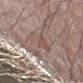notes = catalogued during a skin exam; not biopsied
anatomic site = the left forearm
image source = 15 mm crop, total-body photography
patient = male, aged 53 to 57